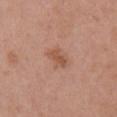Clinical impression:
Part of a total-body skin-imaging series; this lesion was reviewed on a skin check and was not flagged for biopsy.
Clinical summary:
Measured at roughly 3 mm in maximum diameter. Located on the chest. The total-body-photography lesion software estimated an eccentricity of roughly 0.85 and a symmetry-axis asymmetry near 0.25. It also reported an average lesion color of about L≈53 a*≈23 b*≈30 (CIELAB), roughly 9 lightness units darker than nearby skin, and a normalized border contrast of about 6.5. It also reported a border-irregularity rating of about 3/10, a color-variation rating of about 2.5/10, and a peripheral color-asymmetry measure near 1. It also reported a classifier nevus-likeness of about 55/100. This image is a 15 mm lesion crop taken from a total-body photograph. A female subject approximately 65 years of age.